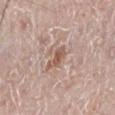Recorded during total-body skin imaging; not selected for excision or biopsy. The subject is a male aged approximately 70. A 15 mm close-up extracted from a 3D total-body photography capture. The lesion is on the right leg.